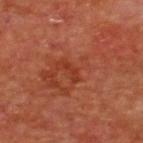notes = catalogued during a skin exam; not biopsied
imaging modality = ~15 mm tile from a whole-body skin photo
location = the back
patient = male, aged 63 to 67
illumination = cross-polarized
automated lesion analysis = an average lesion color of about L≈33 a*≈29 b*≈32 (CIELAB), about 6 CIELAB-L* units darker than the surrounding skin, and a normalized border contrast of about 5.5; a detector confidence of about 100 out of 100 that the crop contains a lesion